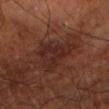workup: imaged on a skin check; not biopsied
size: about 5 mm
illumination: cross-polarized
image source: total-body-photography crop, ~15 mm field of view
patient: male, about 70 years old
anatomic site: the left lower leg